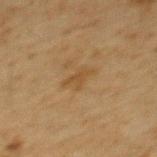Recorded during total-body skin imaging; not selected for excision or biopsy. Imaged with cross-polarized lighting. The recorded lesion diameter is about 3 mm. Cropped from a total-body skin-imaging series; the visible field is about 15 mm. Located on the mid back. A male subject aged 58 to 62.